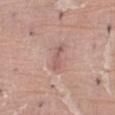This lesion was catalogued during total-body skin photography and was not selected for biopsy. The lesion is located on the abdomen. Automated tile analysis of the lesion measured a border-irregularity index near 5/10 and a peripheral color-asymmetry measure near 0. About 3.5 mm across. Imaged with white-light lighting. A roughly 15 mm field-of-view crop from a total-body skin photograph. A male subject in their 40s.